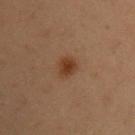Assessment: Captured during whole-body skin photography for melanoma surveillance; the lesion was not biopsied. Background: Automated tile analysis of the lesion measured a lesion area of about 5 mm². The software also gave a lesion color around L≈31 a*≈17 b*≈26 in CIELAB, about 8 CIELAB-L* units darker than the surrounding skin, and a normalized border contrast of about 8. The analysis additionally found a border-irregularity index near 1.5/10, a within-lesion color-variation index near 2.5/10, and radial color variation of about 0.5. From the right upper arm. A female subject, about 40 years old. The tile uses cross-polarized illumination. This image is a 15 mm lesion crop taken from a total-body photograph.